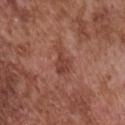The lesion was photographed on a routine skin check and not biopsied; there is no pathology result. The total-body-photography lesion software estimated a footprint of about 5.5 mm², a shape eccentricity near 0.9, and a symmetry-axis asymmetry near 0.5. It also reported about 8 CIELAB-L* units darker than the surrounding skin and a normalized border contrast of about 6.5. The analysis additionally found an automated nevus-likeness rating near 0 out of 100 and a detector confidence of about 100 out of 100 that the crop contains a lesion. Located on the chest. A close-up tile cropped from a whole-body skin photograph, about 15 mm across. A male patient, about 75 years old.